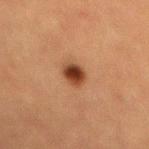  biopsy_status: not biopsied; imaged during a skin examination
  lighting: cross-polarized
  site: mid back
  automated_metrics:
    cielab_L: 33
    cielab_a: 21
    cielab_b: 28
    vs_skin_darker_L: 14.0
    vs_skin_contrast_norm: 12.0
    peripheral_color_asymmetry: 1.5
    nevus_likeness_0_100: 100
    lesion_detection_confidence_0_100: 100
  image:
    source: total-body photography crop
    field_of_view_mm: 15
  patient:
    sex: male
    age_approx: 50
  lesion_size:
    long_diameter_mm_approx: 3.0